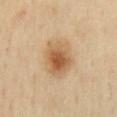Captured during whole-body skin photography for melanoma surveillance; the lesion was not biopsied.